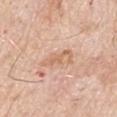Case summary:
– notes — total-body-photography surveillance lesion; no biopsy
– diameter — ~4 mm (longest diameter)
– location — the right upper arm
– subject — male, in their 70s
– imaging modality — ~15 mm crop, total-body skin-cancer survey
– tile lighting — white-light